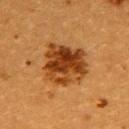follow-up: no biopsy performed (imaged during a skin exam) | site: the upper back | subject: female, aged around 55 | illumination: cross-polarized illumination | image source: 15 mm crop, total-body photography | automated lesion analysis: an average lesion color of about L≈36 a*≈24 b*≈37 (CIELAB), a lesion–skin lightness drop of about 13, and a lesion-to-skin contrast of about 11 (normalized; higher = more distinct); a classifier nevus-likeness of about 95/100 and lesion-presence confidence of about 100/100 | lesion diameter: about 5.5 mm.